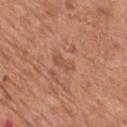Imaged during a routine full-body skin examination; the lesion was not biopsied and no histopathology is available. Captured under white-light illumination. This image is a 15 mm lesion crop taken from a total-body photograph. A male patient aged around 65. On the chest. Longest diameter approximately 3 mm.